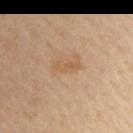The lesion is on the left upper arm. Captured under cross-polarized illumination. The patient is a male in their mid- to late 50s. A 15 mm close-up tile from a total-body photography series done for melanoma screening.